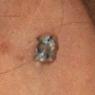Part of a total-body skin-imaging series; this lesion was reviewed on a skin check and was not flagged for biopsy. A 15 mm close-up extracted from a 3D total-body photography capture. The lesion is located on the head or neck. Approximately 6 mm at its widest. A female subject roughly 55 years of age.